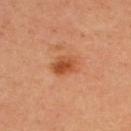notes: catalogued during a skin exam; not biopsied
automated metrics: a border-irregularity index near 2.5/10 and a within-lesion color-variation index near 5/10
patient: female, aged approximately 45
anatomic site: the upper back
lesion size: ≈3.5 mm
image: total-body-photography crop, ~15 mm field of view